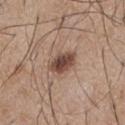biopsy status: imaged on a skin check; not biopsied | tile lighting: white-light | image source: ~15 mm tile from a whole-body skin photo | site: the front of the torso | diameter: ~3.5 mm (longest diameter) | TBP lesion metrics: a footprint of about 6.5 mm² and a shape eccentricity near 0.7; an automated nevus-likeness rating near 95 out of 100 and lesion-presence confidence of about 100/100 | subject: male, aged approximately 55.